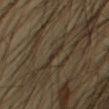notes: no biopsy performed (imaged during a skin exam)
patient: male, aged approximately 45
size: ~2 mm (longest diameter)
lighting: cross-polarized
image: ~15 mm crop, total-body skin-cancer survey
anatomic site: the right upper arm